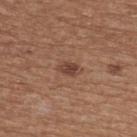A female patient about 75 years old. On the upper back. About 2.5 mm across. A 15 mm close-up extracted from a 3D total-body photography capture.